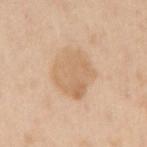No biopsy was performed on this lesion — it was imaged during a full skin examination and was not determined to be concerning. Captured under white-light illumination. A male patient, aged 58–62. From the right upper arm. Cropped from a total-body skin-imaging series; the visible field is about 15 mm. The total-body-photography lesion software estimated a footprint of about 19 mm², a shape eccentricity near 0.45, and a shape-asymmetry score of about 0.15 (0 = symmetric). And it measured a mean CIELAB color near L≈66 a*≈17 b*≈35, a lesion–skin lightness drop of about 8, and a normalized border contrast of about 5.5.